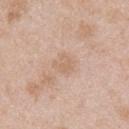The lesion was photographed on a routine skin check and not biopsied; there is no pathology result.
The lesion is on the upper back.
Captured under white-light illumination.
About 2.5 mm across.
Cropped from a whole-body photographic skin survey; the tile spans about 15 mm.
A male subject in their mid- to late 20s.